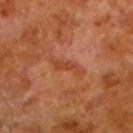Assessment:
The lesion was tiled from a total-body skin photograph and was not biopsied.
Context:
This is a cross-polarized tile. A 15 mm crop from a total-body photograph taken for skin-cancer surveillance. A male patient in their 80s. The lesion-visualizer software estimated a lesion area of about 4.5 mm², a shape eccentricity near 0.95, and a shape-asymmetry score of about 0.4 (0 = symmetric). And it measured roughly 5 lightness units darker than nearby skin and a normalized lesion–skin contrast near 5.5. The analysis additionally found border irregularity of about 5.5 on a 0–10 scale, internal color variation of about 1 on a 0–10 scale, and radial color variation of about 0. From the left lower leg.